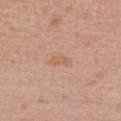Impression:
No biopsy was performed on this lesion — it was imaged during a full skin examination and was not determined to be concerning.
Image and clinical context:
A female subject, about 60 years old. Measured at roughly 3 mm in maximum diameter. The lesion-visualizer software estimated a footprint of about 3 mm² and an eccentricity of roughly 0.9. And it measured a color-variation rating of about 0.5/10 and peripheral color asymmetry of about 0. And it measured an automated nevus-likeness rating near 0 out of 100. A region of skin cropped from a whole-body photographic capture, roughly 15 mm wide. Located on the chest.